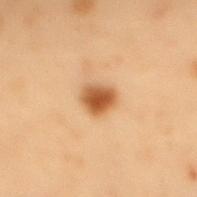Captured during whole-body skin photography for melanoma surveillance; the lesion was not biopsied. The lesion is on the mid back. A male subject, aged around 55. The total-body-photography lesion software estimated a border-irregularity index near 2/10, a within-lesion color-variation index near 4/10, and radial color variation of about 1. The software also gave a detector confidence of about 100 out of 100 that the crop contains a lesion. The recorded lesion diameter is about 3 mm. The tile uses cross-polarized illumination. A close-up tile cropped from a whole-body skin photograph, about 15 mm across.